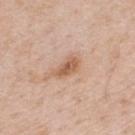workup — imaged on a skin check; not biopsied | image source — total-body-photography crop, ~15 mm field of view | tile lighting — white-light | body site — the upper back | subject — male, aged around 50.